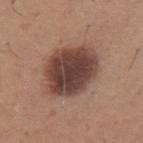Clinical impression: Part of a total-body skin-imaging series; this lesion was reviewed on a skin check and was not flagged for biopsy. Context: The patient is a male about 65 years old. About 7 mm across. A 15 mm close-up tile from a total-body photography series done for melanoma screening. The lesion-visualizer software estimated a border-irregularity index near 1/10 and a color-variation rating of about 5/10. Imaged with white-light lighting. From the upper back.